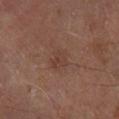Assessment:
Imaged during a routine full-body skin examination; the lesion was not biopsied and no histopathology is available.
Image and clinical context:
This image is a 15 mm lesion crop taken from a total-body photograph. The subject is a male aged 68 to 72. Approximately 3 mm at its widest. The lesion is on the right lower leg. The tile uses cross-polarized illumination.